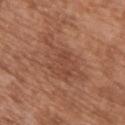The lesion was photographed on a routine skin check and not biopsied; there is no pathology result.
About 8 mm across.
The tile uses white-light illumination.
A male patient, roughly 65 years of age.
The total-body-photography lesion software estimated a lesion area of about 16 mm², an eccentricity of roughly 0.85, and a shape-asymmetry score of about 0.4 (0 = symmetric). The software also gave border irregularity of about 7 on a 0–10 scale and a color-variation rating of about 3/10.
Located on the upper back.
A 15 mm crop from a total-body photograph taken for skin-cancer surveillance.